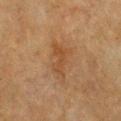Clinical impression:
No biopsy was performed on this lesion — it was imaged during a full skin examination and was not determined to be concerning.
Background:
A 15 mm crop from a total-body photograph taken for skin-cancer surveillance. About 5 mm across. On the chest. A female subject about 55 years old. An algorithmic analysis of the crop reported an average lesion color of about L≈39 a*≈17 b*≈31 (CIELAB), about 6 CIELAB-L* units darker than the surrounding skin, and a lesion-to-skin contrast of about 5.5 (normalized; higher = more distinct). It also reported an automated nevus-likeness rating near 5 out of 100 and a detector confidence of about 100 out of 100 that the crop contains a lesion. Imaged with cross-polarized lighting.